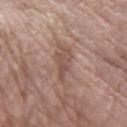Q: Was a biopsy performed?
A: imaged on a skin check; not biopsied